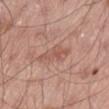{"lighting": "white-light", "site": "left thigh", "patient": {"sex": "male", "age_approx": 55}, "image": {"source": "total-body photography crop", "field_of_view_mm": 15}}